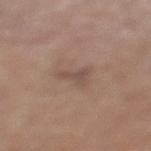Part of a total-body skin-imaging series; this lesion was reviewed on a skin check and was not flagged for biopsy.
On the left lower leg.
A 15 mm close-up tile from a total-body photography series done for melanoma screening.
A male subject, aged around 75.